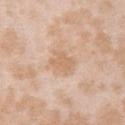Part of a total-body skin-imaging series; this lesion was reviewed on a skin check and was not flagged for biopsy. Imaged with white-light lighting. Cropped from a whole-body photographic skin survey; the tile spans about 15 mm. The subject is a female roughly 25 years of age. The lesion-visualizer software estimated a footprint of about 5.5 mm² and a shape-asymmetry score of about 0.25 (0 = symmetric). The software also gave about 7 CIELAB-L* units darker than the surrounding skin and a lesion-to-skin contrast of about 5.5 (normalized; higher = more distinct). It also reported a border-irregularity rating of about 2.5/10, a within-lesion color-variation index near 1/10, and a peripheral color-asymmetry measure near 0.5. It also reported a classifier nevus-likeness of about 0/100 and a detector confidence of about 100 out of 100 that the crop contains a lesion. The lesion is located on the right upper arm.